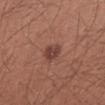{"biopsy_status": "not biopsied; imaged during a skin examination", "automated_metrics": {"border_irregularity_0_10": 1.5, "color_variation_0_10": 2.5, "nevus_likeness_0_100": 70, "lesion_detection_confidence_0_100": 100}, "image": {"source": "total-body photography crop", "field_of_view_mm": 15}, "patient": {"sex": "male", "age_approx": 30}, "site": "right forearm", "lighting": "white-light"}